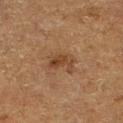Part of a total-body skin-imaging series; this lesion was reviewed on a skin check and was not flagged for biopsy.
The lesion's longest dimension is about 3 mm.
The patient is a male about 75 years old.
From the right lower leg.
An algorithmic analysis of the crop reported a classifier nevus-likeness of about 20/100.
Cropped from a total-body skin-imaging series; the visible field is about 15 mm.
The tile uses cross-polarized illumination.